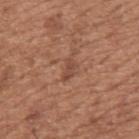{
  "biopsy_status": "not biopsied; imaged during a skin examination",
  "automated_metrics": {
    "cielab_L": 47,
    "cielab_a": 22,
    "cielab_b": 29,
    "vs_skin_darker_L": 8.0
  },
  "image": {
    "source": "total-body photography crop",
    "field_of_view_mm": 15
  },
  "lesion_size": {
    "long_diameter_mm_approx": 2.5
  },
  "lighting": "white-light",
  "patient": {
    "sex": "male",
    "age_approx": 65
  },
  "site": "upper back"
}